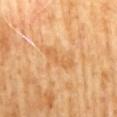Clinical impression: Captured during whole-body skin photography for melanoma surveillance; the lesion was not biopsied.